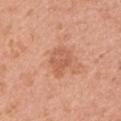A 15 mm close-up extracted from a 3D total-body photography capture. The lesion is located on the right upper arm. A female subject, roughly 40 years of age.